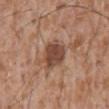This lesion was catalogued during total-body skin photography and was not selected for biopsy.
Longest diameter approximately 4 mm.
A roughly 15 mm field-of-view crop from a total-body skin photograph.
A male patient, about 45 years old.
Located on the front of the torso.